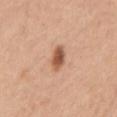Findings:
- notes — imaged on a skin check; not biopsied
- illumination — white-light illumination
- automated lesion analysis — a lesion color around L≈56 a*≈23 b*≈33 in CIELAB and a lesion–skin lightness drop of about 14; border irregularity of about 1.5 on a 0–10 scale, a within-lesion color-variation index near 4.5/10, and a peripheral color-asymmetry measure near 1.5; an automated nevus-likeness rating near 95 out of 100 and a detector confidence of about 100 out of 100 that the crop contains a lesion
- image source — 15 mm crop, total-body photography
- patient — female, roughly 45 years of age
- site — the right upper arm